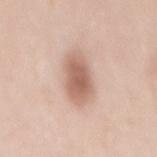biopsy_status: not biopsied; imaged during a skin examination
site: back
image:
  source: total-body photography crop
  field_of_view_mm: 15
patient:
  sex: female
  age_approx: 40
lighting: white-light
automated_metrics:
  area_mm2_approx: 13.0
  eccentricity: 0.9
  shape_asymmetry: 0.15
  cielab_L: 62
  cielab_a: 20
  cielab_b: 27
  vs_skin_darker_L: 13.0
  vs_skin_contrast_norm: 8.0
  border_irregularity_0_10: 2.0
  color_variation_0_10: 3.5
  peripheral_color_asymmetry: 1.0